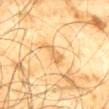Clinical impression: Captured during whole-body skin photography for melanoma surveillance; the lesion was not biopsied. Background: The lesion is located on the mid back. A male patient aged approximately 65. A 15 mm close-up tile from a total-body photography series done for melanoma screening.